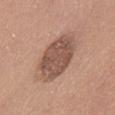Recorded during total-body skin imaging; not selected for excision or biopsy.
Automated image analysis of the tile measured a mean CIELAB color near L≈52 a*≈19 b*≈26, roughly 12 lightness units darker than nearby skin, and a lesion-to-skin contrast of about 8.5 (normalized; higher = more distinct). It also reported a classifier nevus-likeness of about 5/100 and a detector confidence of about 100 out of 100 that the crop contains a lesion.
The subject is a female in their mid- to late 40s.
Located on the abdomen.
This image is a 15 mm lesion crop taken from a total-body photograph.
About 6 mm across.
Imaged with white-light lighting.